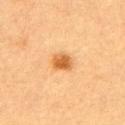Q: Was this lesion biopsied?
A: imaged on a skin check; not biopsied
Q: Lesion location?
A: the upper back
Q: What are the patient's age and sex?
A: female, in their 60s
Q: How was this image acquired?
A: 15 mm crop, total-body photography